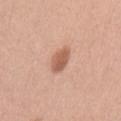workup: no biopsy performed (imaged during a skin exam) | location: the left upper arm | lighting: white-light | subject: female, approximately 35 years of age | imaging modality: ~15 mm crop, total-body skin-cancer survey | automated metrics: a footprint of about 5.5 mm² and a shape-asymmetry score of about 0.2 (0 = symmetric); a border-irregularity rating of about 1.5/10 and a peripheral color-asymmetry measure near 1; a classifier nevus-likeness of about 90/100 and a detector confidence of about 100 out of 100 that the crop contains a lesion | size: ≈3 mm.